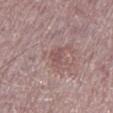Case summary:
* biopsy status — no biopsy performed (imaged during a skin exam)
* location — the left lower leg
* automated metrics — a lesion area of about 3 mm², a shape eccentricity near 0.85, and a symmetry-axis asymmetry near 0.35; a border-irregularity index near 3.5/10, a color-variation rating of about 2/10, and peripheral color asymmetry of about 1
* lesion diameter — ≈2.5 mm
* lighting — white-light illumination
* patient — male, in their mid- to late 70s
* acquisition — total-body-photography crop, ~15 mm field of view